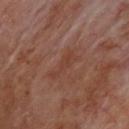biopsy_status: not biopsied; imaged during a skin examination
lighting: cross-polarized
automated_metrics:
  cielab_L: 39
  cielab_a: 22
  cielab_b: 26
  vs_skin_darker_L: 5.0
  vs_skin_contrast_norm: 4.5
  border_irregularity_0_10: 6.0
  color_variation_0_10: 1.0
  peripheral_color_asymmetry: 0.0
  nevus_likeness_0_100: 0
image:
  source: total-body photography crop
  field_of_view_mm: 15
site: upper back
patient:
  sex: male
  age_approx: 70